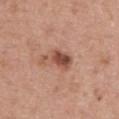Assessment: The lesion was photographed on a routine skin check and not biopsied; there is no pathology result. Clinical summary: The recorded lesion diameter is about 4 mm. Imaged with white-light lighting. Located on the upper back. The total-body-photography lesion software estimated an average lesion color of about L≈50 a*≈24 b*≈29 (CIELAB), roughly 13 lightness units darker than nearby skin, and a normalized lesion–skin contrast near 9. The analysis additionally found internal color variation of about 5 on a 0–10 scale and radial color variation of about 1.5. It also reported a classifier nevus-likeness of about 80/100 and a lesion-detection confidence of about 100/100. A 15 mm close-up extracted from a 3D total-body photography capture. A male patient in their mid-60s.